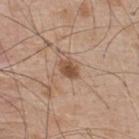The lesion was photographed on a routine skin check and not biopsied; there is no pathology result. The lesion-visualizer software estimated a lesion area of about 4 mm², an eccentricity of roughly 0.75, and a symmetry-axis asymmetry near 0.25. It also reported border irregularity of about 2 on a 0–10 scale, a within-lesion color-variation index near 2/10, and a peripheral color-asymmetry measure near 0.5. It also reported an automated nevus-likeness rating near 85 out of 100 and a detector confidence of about 100 out of 100 that the crop contains a lesion. Cropped from a total-body skin-imaging series; the visible field is about 15 mm. The subject is a male aged 48 to 52. From the upper back. About 2.5 mm across.